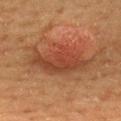biopsy status: imaged on a skin check; not biopsied | lighting: cross-polarized illumination | location: the upper back | lesion size: about 8 mm | patient: male, in their 60s | automated metrics: an area of roughly 24 mm², an eccentricity of roughly 0.85, and a shape-asymmetry score of about 0.25 (0 = symmetric); a lesion color around L≈40 a*≈24 b*≈31 in CIELAB, roughly 9 lightness units darker than nearby skin, and a normalized border contrast of about 7; a color-variation rating of about 5/10 and radial color variation of about 1.5; a nevus-likeness score of about 90/100 | imaging modality: total-body-photography crop, ~15 mm field of view.